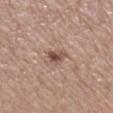{"biopsy_status": "not biopsied; imaged during a skin examination", "lighting": "white-light", "lesion_size": {"long_diameter_mm_approx": 2.5}, "site": "right lower leg", "patient": {"sex": "female", "age_approx": 55}, "image": {"source": "total-body photography crop", "field_of_view_mm": 15}}